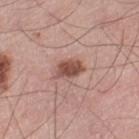workup: catalogued during a skin exam; not biopsied | acquisition: ~15 mm crop, total-body skin-cancer survey | TBP lesion metrics: a footprint of about 5.5 mm², a shape eccentricity near 0.7, and two-axis asymmetry of about 0.2; a border-irregularity index near 2.5/10, a color-variation rating of about 2.5/10, and peripheral color asymmetry of about 1; a classifier nevus-likeness of about 90/100 and lesion-presence confidence of about 100/100 | patient: male, aged 68 to 72 | location: the left thigh.